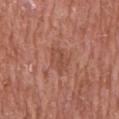Q: Was this lesion biopsied?
A: imaged on a skin check; not biopsied
Q: How was this image acquired?
A: ~15 mm crop, total-body skin-cancer survey
Q: What are the patient's age and sex?
A: male, aged around 65
Q: Lesion location?
A: the chest
Q: Lesion size?
A: ≈3.5 mm
Q: Automated lesion metrics?
A: a footprint of about 5 mm² and two-axis asymmetry of about 0.35; a border-irregularity index near 4/10 and radial color variation of about 1
Q: How was the tile lit?
A: white-light illumination